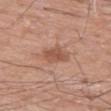A roughly 15 mm field-of-view crop from a total-body skin photograph. The lesion is located on the left thigh. A male patient, aged 58 to 62.